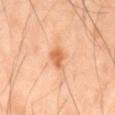Captured during whole-body skin photography for melanoma surveillance; the lesion was not biopsied. The tile uses cross-polarized illumination. An algorithmic analysis of the crop reported a lesion area of about 4.5 mm², an eccentricity of roughly 0.7, and a shape-asymmetry score of about 0.25 (0 = symmetric). The software also gave border irregularity of about 2.5 on a 0–10 scale, a color-variation rating of about 1.5/10, and peripheral color asymmetry of about 0.5. And it measured a nevus-likeness score of about 90/100. A 15 mm close-up extracted from a 3D total-body photography capture. A male patient in their mid- to late 40s. Located on the mid back.